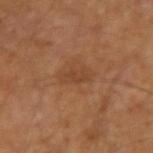{
  "biopsy_status": "not biopsied; imaged during a skin examination",
  "site": "left arm",
  "lesion_size": {
    "long_diameter_mm_approx": 3.0
  },
  "image": {
    "source": "total-body photography crop",
    "field_of_view_mm": 15
  },
  "lighting": "cross-polarized",
  "patient": {
    "sex": "male",
    "age_approx": 65
  }
}